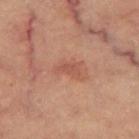{"biopsy_status": "not biopsied; imaged during a skin examination", "image": {"source": "total-body photography crop", "field_of_view_mm": 15}, "lighting": "cross-polarized", "patient": {"sex": "male", "age_approx": 60}, "site": "left thigh", "automated_metrics": {"eccentricity": 0.85, "nevus_likeness_0_100": 10, "lesion_detection_confidence_0_100": 100}}